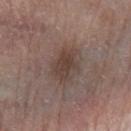Impression:
No biopsy was performed on this lesion — it was imaged during a full skin examination and was not determined to be concerning.
Clinical summary:
About 4.5 mm across. A 15 mm close-up extracted from a 3D total-body photography capture. This is a white-light tile. A female subject, aged around 65. From the left forearm.